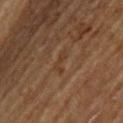{"image": {"source": "total-body photography crop", "field_of_view_mm": 15}, "patient": {"sex": "male", "age_approx": 65}, "site": "upper back", "lesion_size": {"long_diameter_mm_approx": 3.0}, "lighting": "cross-polarized", "automated_metrics": {"area_mm2_approx": 2.5, "eccentricity": 0.9, "shape_asymmetry": 0.6, "cielab_L": 33, "cielab_a": 17, "cielab_b": 28, "vs_skin_darker_L": 4.0, "border_irregularity_0_10": 6.5, "nevus_likeness_0_100": 0}}